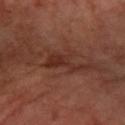biopsy status: catalogued during a skin exam; not biopsied
imaging modality: total-body-photography crop, ~15 mm field of view
lighting: cross-polarized illumination
patient: female, in their mid-60s
size: ≈4.5 mm
body site: the arm